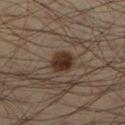Acquisition and patient details: This image is a 15 mm lesion crop taken from a total-body photograph. The subject is a male aged around 35. Longest diameter approximately 3 mm. From the leg.